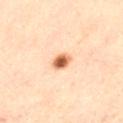follow-up: total-body-photography surveillance lesion; no biopsy
subject: male, aged 53 to 57
body site: the left thigh
illumination: cross-polarized
image source: ~15 mm tile from a whole-body skin photo
automated metrics: a classifier nevus-likeness of about 100/100
lesion size: ~2 mm (longest diameter)